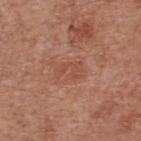Part of a total-body skin-imaging series; this lesion was reviewed on a skin check and was not flagged for biopsy. A lesion tile, about 15 mm wide, cut from a 3D total-body photograph. A male subject, in their mid- to late 60s. Captured under white-light illumination. Longest diameter approximately 3 mm. Automated image analysis of the tile measured an average lesion color of about L≈50 a*≈25 b*≈31 (CIELAB), about 6 CIELAB-L* units darker than the surrounding skin, and a lesion-to-skin contrast of about 4.5 (normalized; higher = more distinct). The software also gave border irregularity of about 6 on a 0–10 scale, a within-lesion color-variation index near 1.5/10, and radial color variation of about 0.5. On the upper back.